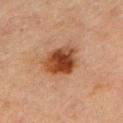workup = catalogued during a skin exam; not biopsied
patient = male, aged 63 to 67
lesion size = about 4.5 mm
imaging modality = ~15 mm crop, total-body skin-cancer survey
anatomic site = the right upper arm
tile lighting = cross-polarized